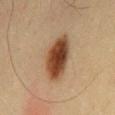workup: no biopsy performed (imaged during a skin exam) | diameter: about 6 mm | illumination: cross-polarized | subject: male, roughly 35 years of age | image source: ~15 mm crop, total-body skin-cancer survey | site: the mid back | TBP lesion metrics: a shape eccentricity near 0.85 and two-axis asymmetry of about 0.2.